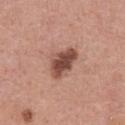Notes:
* patient · male, aged 53 to 57
* image · 15 mm crop, total-body photography
* body site · the chest
* illumination · white-light
* automated lesion analysis · a lesion area of about 9 mm²; border irregularity of about 2.5 on a 0–10 scale and peripheral color asymmetry of about 1.5; a detector confidence of about 100 out of 100 that the crop contains a lesion